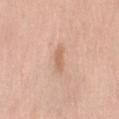Part of a total-body skin-imaging series; this lesion was reviewed on a skin check and was not flagged for biopsy.
This is a white-light tile.
The lesion is located on the left upper arm.
A female patient, aged 63 to 67.
A close-up tile cropped from a whole-body skin photograph, about 15 mm across.
The recorded lesion diameter is about 2.5 mm.